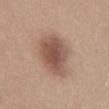Clinical impression:
Imaged during a routine full-body skin examination; the lesion was not biopsied and no histopathology is available.
Image and clinical context:
A female subject aged approximately 30. Located on the back. Cropped from a whole-body photographic skin survey; the tile spans about 15 mm. The lesion-visualizer software estimated a lesion area of about 20 mm² and a shape-asymmetry score of about 0.2 (0 = symmetric). The analysis additionally found a lesion color around L≈53 a*≈19 b*≈26 in CIELAB. This is a white-light tile.